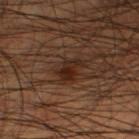Assessment:
The lesion was tiled from a total-body skin photograph and was not biopsied.
Image and clinical context:
A close-up tile cropped from a whole-body skin photograph, about 15 mm across. Captured under cross-polarized illumination. Longest diameter approximately 3 mm. Automated image analysis of the tile measured a border-irregularity index near 4.5/10, internal color variation of about 3.5 on a 0–10 scale, and a peripheral color-asymmetry measure near 1. The software also gave a classifier nevus-likeness of about 90/100 and a detector confidence of about 100 out of 100 that the crop contains a lesion. The subject is a male aged approximately 45. The lesion is located on the right thigh.